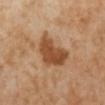No biopsy was performed on this lesion — it was imaged during a full skin examination and was not determined to be concerning.
From the left lower leg.
A 15 mm close-up tile from a total-body photography series done for melanoma screening.
A female subject, approximately 50 years of age.
Approximately 5 mm at its widest.
The tile uses cross-polarized illumination.